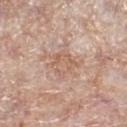Impression: Recorded during total-body skin imaging; not selected for excision or biopsy. Background: A 15 mm close-up tile from a total-body photography series done for melanoma screening. The lesion is located on the right lower leg. A female patient roughly 70 years of age.